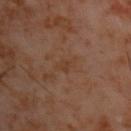The lesion was tiled from a total-body skin photograph and was not biopsied.
Approximately 3 mm at its widest.
A lesion tile, about 15 mm wide, cut from a 3D total-body photograph.
The lesion-visualizer software estimated a mean CIELAB color near L≈35 a*≈18 b*≈27, roughly 4 lightness units darker than nearby skin, and a normalized border contrast of about 4.5. And it measured a border-irregularity index near 3/10, internal color variation of about 1.5 on a 0–10 scale, and radial color variation of about 0.5. It also reported a nevus-likeness score of about 0/100 and a detector confidence of about 100 out of 100 that the crop contains a lesion.
A male subject, aged approximately 60.
Located on the upper back.
Imaged with cross-polarized lighting.